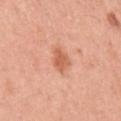Case summary:
- workup · catalogued during a skin exam; not biopsied
- automated metrics · an eccentricity of roughly 0.55 and two-axis asymmetry of about 0.3; a lesion color around L≈61 a*≈28 b*≈35 in CIELAB, about 10 CIELAB-L* units darker than the surrounding skin, and a normalized border contrast of about 7; an automated nevus-likeness rating near 60 out of 100
- lesion diameter · about 2.5 mm
- site · the left upper arm
- subject · female, aged 53 to 57
- imaging modality · ~15 mm crop, total-body skin-cancer survey
- illumination · white-light